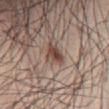Assessment:
This lesion was catalogued during total-body skin photography and was not selected for biopsy.
Clinical summary:
The total-body-photography lesion software estimated a lesion color around L≈45 a*≈18 b*≈23 in CIELAB and a normalized lesion–skin contrast near 9.5. The analysis additionally found a border-irregularity index near 3/10, a color-variation rating of about 3.5/10, and radial color variation of about 1. It also reported a nevus-likeness score of about 85/100 and a lesion-detection confidence of about 100/100. Cropped from a total-body skin-imaging series; the visible field is about 15 mm. About 3 mm across. Captured under white-light illumination. A male patient roughly 25 years of age. From the abdomen.